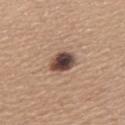The lesion was tiled from a total-body skin photograph and was not biopsied. From the upper back. A female subject, approximately 55 years of age. This image is a 15 mm lesion crop taken from a total-body photograph. Measured at roughly 3.5 mm in maximum diameter. Imaged with white-light lighting.